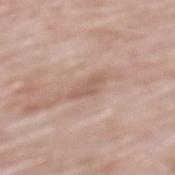Impression: Captured during whole-body skin photography for melanoma surveillance; the lesion was not biopsied. Context: Measured at roughly 3.5 mm in maximum diameter. The lesion-visualizer software estimated a border-irregularity rating of about 3.5/10, internal color variation of about 0.5 on a 0–10 scale, and peripheral color asymmetry of about 0.5. The software also gave a nevus-likeness score of about 0/100. A male patient aged approximately 80. On the mid back. Imaged with white-light lighting. A region of skin cropped from a whole-body photographic capture, roughly 15 mm wide.